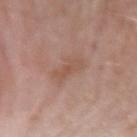Recorded during total-body skin imaging; not selected for excision or biopsy.